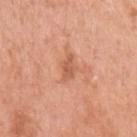The lesion was photographed on a routine skin check and not biopsied; there is no pathology result. A region of skin cropped from a whole-body photographic capture, roughly 15 mm wide. On the left upper arm. A female subject, aged 63 to 67. The lesion's longest dimension is about 3.5 mm.